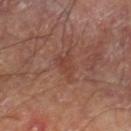The lesion was tiled from a total-body skin photograph and was not biopsied. A male subject roughly 70 years of age. From the right lower leg. A 15 mm crop from a total-body photograph taken for skin-cancer surveillance. This is a cross-polarized tile.